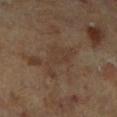Q: Was a biopsy performed?
A: imaged on a skin check; not biopsied
Q: What is the lesion's diameter?
A: ≈5 mm
Q: What is the imaging modality?
A: total-body-photography crop, ~15 mm field of view
Q: What is the anatomic site?
A: the left leg
Q: How was the tile lit?
A: cross-polarized
Q: What are the patient's age and sex?
A: female, aged 63–67